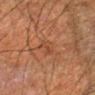notes = total-body-photography surveillance lesion; no biopsy | image source = total-body-photography crop, ~15 mm field of view | subject = male, aged 48–52 | body site = the left forearm.